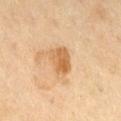Impression: The lesion was tiled from a total-body skin photograph and was not biopsied. Context: A male patient, aged approximately 70. A lesion tile, about 15 mm wide, cut from a 3D total-body photograph. From the right thigh. Longest diameter approximately 3.5 mm. Captured under cross-polarized illumination.